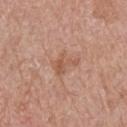Q: Was this lesion biopsied?
A: no biopsy performed (imaged during a skin exam)
Q: What are the patient's age and sex?
A: male, aged 68–72
Q: What did automated image analysis measure?
A: a lesion color around L≈56 a*≈22 b*≈30 in CIELAB and a lesion-to-skin contrast of about 5.5 (normalized; higher = more distinct); border irregularity of about 4.5 on a 0–10 scale and a within-lesion color-variation index near 3/10; an automated nevus-likeness rating near 0 out of 100
Q: Lesion location?
A: the right upper arm
Q: What lighting was used for the tile?
A: white-light
Q: What kind of image is this?
A: total-body-photography crop, ~15 mm field of view